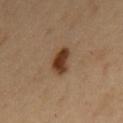notes: imaged on a skin check; not biopsied | lighting: cross-polarized | acquisition: ~15 mm tile from a whole-body skin photo | anatomic site: the mid back | TBP lesion metrics: a nevus-likeness score of about 100/100 and a lesion-detection confidence of about 100/100 | subject: male, about 50 years old.